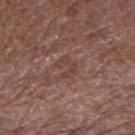Assessment:
The lesion was tiled from a total-body skin photograph and was not biopsied.
Context:
This image is a 15 mm lesion crop taken from a total-body photograph. From the right forearm. The recorded lesion diameter is about 2.5 mm. A male patient aged 43–47.